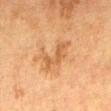Assessment:
This lesion was catalogued during total-body skin photography and was not selected for biopsy.
Clinical summary:
Automated tile analysis of the lesion measured an area of roughly 5.5 mm², a shape eccentricity near 0.95, and a symmetry-axis asymmetry near 0.5. The analysis additionally found a nevus-likeness score of about 0/100. A 15 mm close-up extracted from a 3D total-body photography capture. The lesion is located on the abdomen. Captured under cross-polarized illumination. About 4.5 mm across. The patient is a female aged around 50.